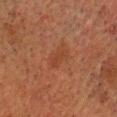notes: imaged on a skin check; not biopsied | lighting: cross-polarized illumination | image: total-body-photography crop, ~15 mm field of view | site: the head or neck | lesion diameter: ~3 mm (longest diameter) | subject: male, in their 60s | automated metrics: an area of roughly 2.5 mm², an eccentricity of roughly 0.95, and a shape-asymmetry score of about 0.35 (0 = symmetric); a nevus-likeness score of about 0/100 and a detector confidence of about 100 out of 100 that the crop contains a lesion.